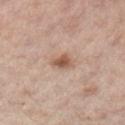notes: total-body-photography surveillance lesion; no biopsy | TBP lesion metrics: an average lesion color of about L≈45 a*≈16 b*≈24 (CIELAB), a lesion–skin lightness drop of about 9, and a normalized border contrast of about 8; a border-irregularity index near 2/10, a color-variation rating of about 2.5/10, and peripheral color asymmetry of about 1 | image: 15 mm crop, total-body photography | location: the left upper arm | subject: female, roughly 55 years of age | lesion diameter: ~2.5 mm (longest diameter) | illumination: cross-polarized illumination.